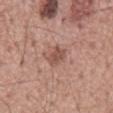follow-up: no biopsy performed (imaged during a skin exam)